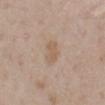image source=total-body-photography crop, ~15 mm field of view
subject=female, aged around 30
anatomic site=the mid back
lighting=white-light illumination
lesion size=~3 mm (longest diameter)